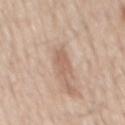Imaged during a routine full-body skin examination; the lesion was not biopsied and no histopathology is available.
From the back.
A male subject about 60 years old.
A lesion tile, about 15 mm wide, cut from a 3D total-body photograph.
The tile uses white-light illumination.